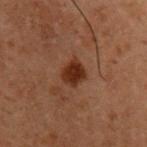Clinical impression:
Captured during whole-body skin photography for melanoma surveillance; the lesion was not biopsied.
Acquisition and patient details:
From the chest. The recorded lesion diameter is about 3 mm. A 15 mm close-up tile from a total-body photography series done for melanoma screening. The tile uses cross-polarized illumination. A male patient, approximately 60 years of age.